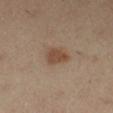This lesion was catalogued during total-body skin photography and was not selected for biopsy. The lesion's longest dimension is about 3 mm. From the left leg. The patient is a female aged around 40. An algorithmic analysis of the crop reported a within-lesion color-variation index near 1.5/10 and peripheral color asymmetry of about 0.5. A 15 mm close-up extracted from a 3D total-body photography capture. The tile uses cross-polarized illumination.